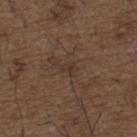A male patient, aged approximately 50.
Approximately 3 mm at its widest.
Imaged with white-light lighting.
This image is a 15 mm lesion crop taken from a total-body photograph.
On the upper back.
The total-body-photography lesion software estimated about 5 CIELAB-L* units darker than the surrounding skin and a lesion-to-skin contrast of about 5 (normalized; higher = more distinct). The analysis additionally found a border-irregularity rating of about 5/10, a within-lesion color-variation index near 0.5/10, and radial color variation of about 0.